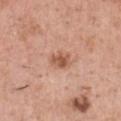{"biopsy_status": "not biopsied; imaged during a skin examination", "site": "chest", "lighting": "white-light", "image": {"source": "total-body photography crop", "field_of_view_mm": 15}, "lesion_size": {"long_diameter_mm_approx": 3.0}, "automated_metrics": {"border_irregularity_0_10": 2.5, "color_variation_0_10": 3.5, "peripheral_color_asymmetry": 1.0}, "patient": {"sex": "male", "age_approx": 45}}